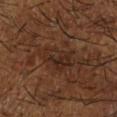Q: Is there a histopathology result?
A: total-body-photography surveillance lesion; no biopsy